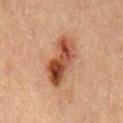No biopsy was performed on this lesion — it was imaged during a full skin examination and was not determined to be concerning.
On the mid back.
An algorithmic analysis of the crop reported an average lesion color of about L≈40 a*≈22 b*≈29 (CIELAB) and a lesion–skin lightness drop of about 13. It also reported a within-lesion color-variation index near 9/10 and radial color variation of about 3.
A 15 mm close-up tile from a total-body photography series done for melanoma screening.
The recorded lesion diameter is about 6.5 mm.
A male patient, approximately 70 years of age.